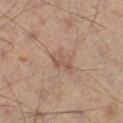This image is a 15 mm lesion crop taken from a total-body photograph. An algorithmic analysis of the crop reported a border-irregularity index near 5/10, internal color variation of about 2 on a 0–10 scale, and radial color variation of about 1. It also reported an automated nevus-likeness rating near 5 out of 100. The lesion's longest dimension is about 3 mm. A male patient, aged around 50. From the left leg. Imaged with cross-polarized lighting.